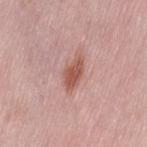follow-up = imaged on a skin check; not biopsied | image source = ~15 mm crop, total-body skin-cancer survey | lesion diameter = ≈4 mm | illumination = white-light | subject = female, aged around 50 | site = the left thigh | automated metrics = a lesion color around L≈55 a*≈24 b*≈27 in CIELAB, a lesion–skin lightness drop of about 11, and a normalized border contrast of about 8.5; a border-irregularity index near 2/10, a color-variation rating of about 2/10, and a peripheral color-asymmetry measure near 0.5; a nevus-likeness score of about 75/100 and a detector confidence of about 100 out of 100 that the crop contains a lesion.